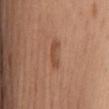Recorded during total-body skin imaging; not selected for excision or biopsy. A lesion tile, about 15 mm wide, cut from a 3D total-body photograph. The subject is a female aged 63 to 67. Approximately 3.5 mm at its widest. The lesion is on the front of the torso. Imaged with white-light lighting.